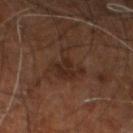notes — imaged on a skin check; not biopsied
diameter — about 4 mm
imaging modality — 15 mm crop, total-body photography
location — the right leg
patient — male, approximately 60 years of age